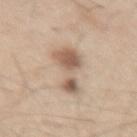follow-up: no biopsy performed (imaged during a skin exam)
image-analysis metrics: a lesion-to-skin contrast of about 8 (normalized; higher = more distinct); border irregularity of about 6.5 on a 0–10 scale and radial color variation of about 1.5
tile lighting: white-light illumination
body site: the right thigh
image source: ~15 mm crop, total-body skin-cancer survey
subject: male, aged 58 to 62
diameter: ~6.5 mm (longest diameter)